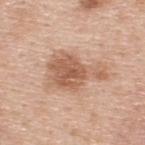diameter: about 6.5 mm; location: the upper back; subject: male, in their 50s; illumination: white-light illumination; acquisition: ~15 mm crop, total-body skin-cancer survey.